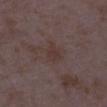Assessment: Imaged during a routine full-body skin examination; the lesion was not biopsied and no histopathology is available. Clinical summary: This image is a 15 mm lesion crop taken from a total-body photograph. Automated image analysis of the tile measured roughly 5 lightness units darker than nearby skin and a normalized lesion–skin contrast near 5.5. It also reported internal color variation of about 1.5 on a 0–10 scale and peripheral color asymmetry of about 0.5. The analysis additionally found an automated nevus-likeness rating near 0 out of 100 and a lesion-detection confidence of about 100/100. From the right lower leg. A female patient, in their mid- to late 30s.